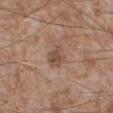lesion diameter: about 2.5 mm
site: the right lower leg
patient: male, approximately 65 years of age
image source: ~15 mm crop, total-body skin-cancer survey
lighting: white-light illumination
automated lesion analysis: a footprint of about 5.5 mm², an outline eccentricity of about 0.5 (0 = round, 1 = elongated), and two-axis asymmetry of about 0.2; a border-irregularity index near 2/10 and a peripheral color-asymmetry measure near 1; a nevus-likeness score of about 0/100 and a detector confidence of about 100 out of 100 that the crop contains a lesion